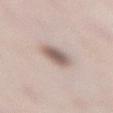The lesion was tiled from a total-body skin photograph and was not biopsied.
Cropped from a whole-body photographic skin survey; the tile spans about 15 mm.
On the lower back.
A female subject, about 50 years old.